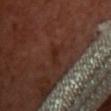No biopsy was performed on this lesion — it was imaged during a full skin examination and was not determined to be concerning. The lesion is located on the chest. A lesion tile, about 15 mm wide, cut from a 3D total-body photograph. A female patient, in their mid- to late 50s.